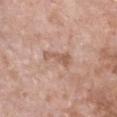Case summary:
* subject — female, aged approximately 75
* acquisition — ~15 mm tile from a whole-body skin photo
* lesion diameter — ~4 mm (longest diameter)
* tile lighting — white-light
* anatomic site — the front of the torso